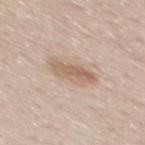Q: Was a biopsy performed?
A: imaged on a skin check; not biopsied
Q: Patient demographics?
A: male, aged 58–62
Q: Lesion size?
A: ≈4.5 mm
Q: What is the imaging modality?
A: ~15 mm crop, total-body skin-cancer survey
Q: What is the anatomic site?
A: the mid back